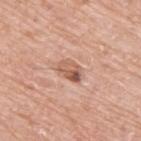biopsy_status: not biopsied; imaged during a skin examination
site: upper back
image:
  source: total-body photography crop
  field_of_view_mm: 15
patient:
  sex: male
  age_approx: 75